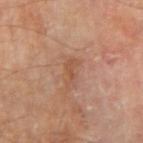The lesion is on the right upper arm. Cropped from a whole-body photographic skin survey; the tile spans about 15 mm. Imaged with cross-polarized lighting. The patient is a male roughly 65 years of age.